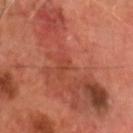biopsy_status: not biopsied; imaged during a skin examination
automated_metrics:
  eccentricity: 0.9
  shape_asymmetry: 0.5
  color_variation_0_10: 8.5
  peripheral_color_asymmetry: 3.0
lighting: cross-polarized
image:
  source: total-body photography crop
  field_of_view_mm: 15
patient:
  sex: male
  age_approx: 70
lesion_size:
  long_diameter_mm_approx: 13.0
site: head or neck